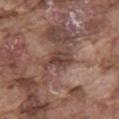Findings:
- notes: imaged on a skin check; not biopsied
- subject: male, aged approximately 75
- size: about 4 mm
- location: the mid back
- imaging modality: ~15 mm crop, total-body skin-cancer survey